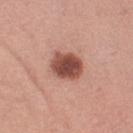workup — no biopsy performed (imaged during a skin exam) | subject — female, aged approximately 55 | image source — ~15 mm crop, total-body skin-cancer survey | body site — the left thigh | TBP lesion metrics — a footprint of about 11 mm², a shape eccentricity near 0.6, and a shape-asymmetry score of about 0.15 (0 = symmetric); a border-irregularity index near 1.5/10, internal color variation of about 4.5 on a 0–10 scale, and peripheral color asymmetry of about 1; a nevus-likeness score of about 85/100 and a detector confidence of about 100 out of 100 that the crop contains a lesion.